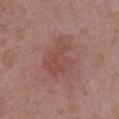workup: total-body-photography surveillance lesion; no biopsy | imaging modality: ~15 mm crop, total-body skin-cancer survey | subject: female, roughly 35 years of age | lesion diameter: ~5 mm (longest diameter) | body site: the chest | illumination: white-light | TBP lesion metrics: an area of roughly 14 mm² and an eccentricity of roughly 0.65; an average lesion color of about L≈48 a*≈24 b*≈24 (CIELAB), about 6 CIELAB-L* units darker than the surrounding skin, and a lesion-to-skin contrast of about 5 (normalized; higher = more distinct); a nevus-likeness score of about 5/100.